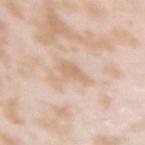<lesion>
<biopsy_status>not biopsied; imaged during a skin examination</biopsy_status>
<lighting>white-light</lighting>
<image>
  <source>total-body photography crop</source>
  <field_of_view_mm>15</field_of_view_mm>
</image>
<site>right upper arm</site>
<patient>
  <sex>female</sex>
  <age_approx>25</age_approx>
</patient>
<lesion_size>
  <long_diameter_mm_approx>3.0</long_diameter_mm_approx>
</lesion_size>
</lesion>